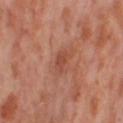  image:
    source: total-body photography crop
    field_of_view_mm: 15
  site: right thigh
  patient:
    sex: female
    age_approx: 55